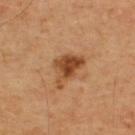biopsy status — no biopsy performed (imaged during a skin exam) | patient — male, in their 50s | site — the upper back | imaging modality — ~15 mm tile from a whole-body skin photo.